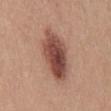<tbp_lesion>
  <biopsy_status>not biopsied; imaged during a skin examination</biopsy_status>
  <patient>
    <sex>male</sex>
    <age_approx>30</age_approx>
  </patient>
  <image>
    <source>total-body photography crop</source>
    <field_of_view_mm>15</field_of_view_mm>
  </image>
  <automated_metrics>
    <eccentricity>0.85</eccentricity>
    <border_irregularity_0_10>2.5</border_irregularity_0_10>
    <color_variation_0_10>8.0</color_variation_0_10>
    <peripheral_color_asymmetry>3.0</peripheral_color_asymmetry>
  </automated_metrics>
  <site>mid back</site>
  <lesion_size>
    <long_diameter_mm_approx>7.0</long_diameter_mm_approx>
  </lesion_size>
</tbp_lesion>